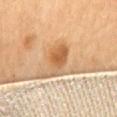Clinical impression: The lesion was tiled from a total-body skin photograph and was not biopsied. Clinical summary: Located on the upper back. The recorded lesion diameter is about 4.5 mm. The subject is a female about 65 years old. Cropped from a total-body skin-imaging series; the visible field is about 15 mm. This is a cross-polarized tile.